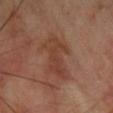  biopsy_status: not biopsied; imaged during a skin examination
  patient:
    sex: female
    age_approx: 55
  lighting: cross-polarized
  site: right forearm
  lesion_size:
    long_diameter_mm_approx: 7.0
  image:
    source: total-body photography crop
    field_of_view_mm: 15